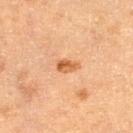<case>
  <biopsy_status>not biopsied; imaged during a skin examination</biopsy_status>
  <patient>
    <sex>female</sex>
    <age_approx>55</age_approx>
  </patient>
  <image>
    <source>total-body photography crop</source>
    <field_of_view_mm>15</field_of_view_mm>
  </image>
  <site>leg</site>
</case>